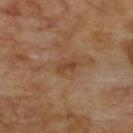The lesion was photographed on a routine skin check and not biopsied; there is no pathology result. On the upper back. Automated tile analysis of the lesion measured a lesion area of about 2.5 mm², an eccentricity of roughly 0.9, and a shape-asymmetry score of about 0.35 (0 = symmetric). The software also gave border irregularity of about 3.5 on a 0–10 scale, a color-variation rating of about 0/10, and radial color variation of about 0. Measured at roughly 2.5 mm in maximum diameter. The tile uses cross-polarized illumination. The subject is a male aged around 70. A close-up tile cropped from a whole-body skin photograph, about 15 mm across.